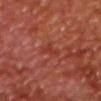Part of a total-body skin-imaging series; this lesion was reviewed on a skin check and was not flagged for biopsy.
An algorithmic analysis of the crop reported a mean CIELAB color near L≈38 a*≈32 b*≈31, about 5 CIELAB-L* units darker than the surrounding skin, and a lesion-to-skin contrast of about 5 (normalized; higher = more distinct). The software also gave a lesion-detection confidence of about 100/100.
The recorded lesion diameter is about 2.5 mm.
The patient is a male in their mid-60s.
Cropped from a total-body skin-imaging series; the visible field is about 15 mm.
Captured under cross-polarized illumination.